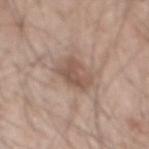notes: imaged on a skin check; not biopsied
image source: ~15 mm tile from a whole-body skin photo
location: the mid back
size: ≈3.5 mm
subject: male, in their mid- to late 40s